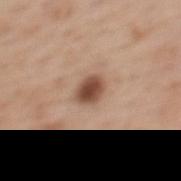Q: Is there a histopathology result?
A: imaged on a skin check; not biopsied
Q: What are the patient's age and sex?
A: female, roughly 45 years of age
Q: What is the anatomic site?
A: the mid back
Q: What kind of image is this?
A: ~15 mm tile from a whole-body skin photo